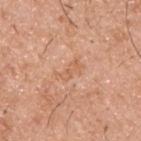Notes:
- workup · total-body-photography surveillance lesion; no biopsy
- imaging modality · total-body-photography crop, ~15 mm field of view
- patient · male, aged 38 to 42
- site · the upper back